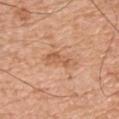  biopsy_status: not biopsied; imaged during a skin examination
  site: chest
  lesion_size:
    long_diameter_mm_approx: 4.0
  lighting: white-light
  image:
    source: total-body photography crop
    field_of_view_mm: 15
  patient:
    sex: male
    age_approx: 75